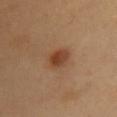Clinical summary:
Captured under cross-polarized illumination. A female subject aged approximately 40. Located on the front of the torso. Automated image analysis of the tile measured roughly 10 lightness units darker than nearby skin and a lesion-to-skin contrast of about 8 (normalized; higher = more distinct). And it measured a border-irregularity rating of about 2/10, a color-variation rating of about 3.5/10, and peripheral color asymmetry of about 1. It also reported a nevus-likeness score of about 100/100. Measured at roughly 3 mm in maximum diameter. A 15 mm close-up tile from a total-body photography series done for melanoma screening.